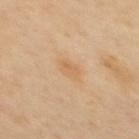• workup — no biopsy performed (imaged during a skin exam)
• subject — female, aged approximately 70
• anatomic site — the upper back
• TBP lesion metrics — a footprint of about 4.5 mm² and a symmetry-axis asymmetry near 0.15; a color-variation rating of about 2.5/10 and radial color variation of about 1; an automated nevus-likeness rating near 10 out of 100 and lesion-presence confidence of about 100/100
• image source — total-body-photography crop, ~15 mm field of view
• tile lighting — cross-polarized illumination